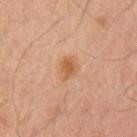The lesion was photographed on a routine skin check and not biopsied; there is no pathology result. An algorithmic analysis of the crop reported a footprint of about 5 mm², an eccentricity of roughly 0.7, and two-axis asymmetry of about 0.25. And it measured roughly 7 lightness units darker than nearby skin and a lesion-to-skin contrast of about 7 (normalized; higher = more distinct). The software also gave a nevus-likeness score of about 90/100 and a lesion-detection confidence of about 100/100. The lesion is located on the left upper arm. A male subject roughly 50 years of age. A lesion tile, about 15 mm wide, cut from a 3D total-body photograph. About 3 mm across. The tile uses cross-polarized illumination.